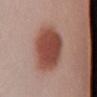{"automated_metrics": {"eccentricity": 0.45, "shape_asymmetry": 0.15, "border_irregularity_0_10": 1.5, "color_variation_0_10": 4.5, "lesion_detection_confidence_0_100": 100}, "site": "left lower leg", "image": {"source": "total-body photography crop", "field_of_view_mm": 15}, "patient": {"sex": "female", "age_approx": 20}, "lesion_size": {"long_diameter_mm_approx": 6.0}}